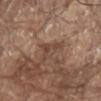subject — male, approximately 80 years of age
acquisition — 15 mm crop, total-body photography
anatomic site — the abdomen
illumination — white-light illumination